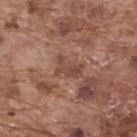{"biopsy_status": "not biopsied; imaged during a skin examination", "patient": {"sex": "male", "age_approx": 75}, "automated_metrics": {"area_mm2_approx": 4.5, "shape_asymmetry": 0.5, "cielab_L": 45, "cielab_a": 21, "cielab_b": 26, "vs_skin_darker_L": 8.0, "vs_skin_contrast_norm": 6.5}, "lesion_size": {"long_diameter_mm_approx": 3.0}, "lighting": "white-light", "image": {"source": "total-body photography crop", "field_of_view_mm": 15}, "site": "upper back"}